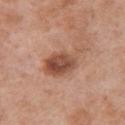The lesion was photographed on a routine skin check and not biopsied; there is no pathology result.